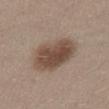No biopsy was performed on this lesion — it was imaged during a full skin examination and was not determined to be concerning.
A male patient, approximately 30 years of age.
On the back.
This image is a 15 mm lesion crop taken from a total-body photograph.